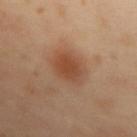Q: Is there a histopathology result?
A: no biopsy performed (imaged during a skin exam)
Q: Patient demographics?
A: female, roughly 55 years of age
Q: How was this image acquired?
A: 15 mm crop, total-body photography
Q: Automated lesion metrics?
A: a lesion color around L≈48 a*≈22 b*≈33 in CIELAB, a lesion–skin lightness drop of about 9, and a lesion-to-skin contrast of about 7 (normalized; higher = more distinct); a classifier nevus-likeness of about 100/100
Q: Where on the body is the lesion?
A: the mid back
Q: How large is the lesion?
A: ≈4 mm